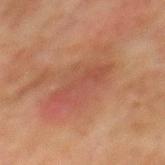The lesion was tiled from a total-body skin photograph and was not biopsied. Approximately 6 mm at its widest. A 15 mm close-up extracted from a 3D total-body photography capture. Imaged with cross-polarized lighting. The lesion is on the mid back. A male patient in their mid- to late 70s.